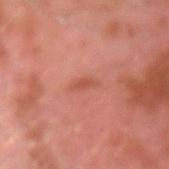Q: Was a biopsy performed?
A: no biopsy performed (imaged during a skin exam)
Q: Where on the body is the lesion?
A: the arm
Q: What is the imaging modality?
A: 15 mm crop, total-body photography
Q: What are the patient's age and sex?
A: male, approximately 30 years of age
Q: Lesion size?
A: ≈2.5 mm
Q: How was the tile lit?
A: cross-polarized